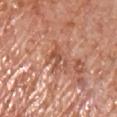– biopsy status — imaged on a skin check; not biopsied
– site — the chest
– acquisition — ~15 mm tile from a whole-body skin photo
– subject — male, aged approximately 65